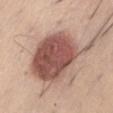biopsy status: catalogued during a skin exam; not biopsied
location: the abdomen
image: ~15 mm tile from a whole-body skin photo
tile lighting: white-light
automated metrics: a footprint of about 34 mm², an eccentricity of roughly 0.7, and a shape-asymmetry score of about 0.2 (0 = symmetric); lesion-presence confidence of about 100/100
lesion diameter: ~9 mm (longest diameter)
subject: male, roughly 35 years of age